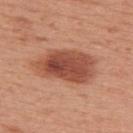Recorded during total-body skin imaging; not selected for excision or biopsy. A 15 mm crop from a total-body photograph taken for skin-cancer surveillance. An algorithmic analysis of the crop reported a footprint of about 21 mm² and a shape eccentricity near 0.85. It also reported an average lesion color of about L≈49 a*≈27 b*≈31 (CIELAB), about 14 CIELAB-L* units darker than the surrounding skin, and a normalized lesion–skin contrast near 9.5. It also reported a border-irregularity index near 2/10 and internal color variation of about 5.5 on a 0–10 scale. The software also gave an automated nevus-likeness rating near 95 out of 100 and a lesion-detection confidence of about 100/100. About 7 mm across. This is a white-light tile. A female patient, in their 50s. On the upper back.